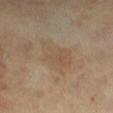follow-up — catalogued during a skin exam; not biopsied | site — the leg | patient — aged 58 to 62 | acquisition — total-body-photography crop, ~15 mm field of view.